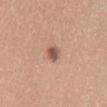Q: Is there a histopathology result?
A: total-body-photography surveillance lesion; no biopsy
Q: What are the patient's age and sex?
A: female, aged 28–32
Q: What did automated image analysis measure?
A: a mean CIELAB color near L≈54 a*≈22 b*≈27, roughly 13 lightness units darker than nearby skin, and a lesion-to-skin contrast of about 8.5 (normalized; higher = more distinct)
Q: How was this image acquired?
A: 15 mm crop, total-body photography
Q: How was the tile lit?
A: white-light
Q: Lesion location?
A: the back
Q: Lesion size?
A: ~2.5 mm (longest diameter)